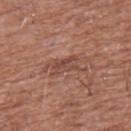Clinical impression:
Captured during whole-body skin photography for melanoma surveillance; the lesion was not biopsied.
Acquisition and patient details:
Approximately 3 mm at its widest. The tile uses white-light illumination. Cropped from a total-body skin-imaging series; the visible field is about 15 mm. A male patient in their 60s. The lesion is on the chest.